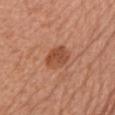notes: imaged on a skin check; not biopsied | diameter: ≈3.5 mm | tile lighting: white-light | body site: the chest | imaging modality: ~15 mm crop, total-body skin-cancer survey | patient: female, aged around 65.